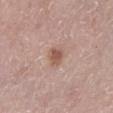This is a white-light tile. Longest diameter approximately 2.5 mm. The lesion is on the leg. This image is a 15 mm lesion crop taken from a total-body photograph. The patient is a female roughly 65 years of age.